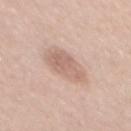biopsy_status: not biopsied; imaged during a skin examination
patient:
  sex: male
  age_approx: 35
lighting: white-light
lesion_size:
  long_diameter_mm_approx: 5.0
site: mid back
automated_metrics:
  vs_skin_darker_L: 10.0
  vs_skin_contrast_norm: 6.0
image:
  source: total-body photography crop
  field_of_view_mm: 15